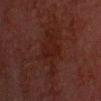Clinical impression:
This lesion was catalogued during total-body skin photography and was not selected for biopsy.